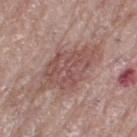Q: Is there a histopathology result?
A: total-body-photography surveillance lesion; no biopsy
Q: How large is the lesion?
A: ≈7 mm
Q: What is the imaging modality?
A: ~15 mm tile from a whole-body skin photo
Q: Automated lesion metrics?
A: an average lesion color of about L≈51 a*≈20 b*≈22 (CIELAB) and about 9 CIELAB-L* units darker than the surrounding skin; border irregularity of about 4.5 on a 0–10 scale, internal color variation of about 3 on a 0–10 scale, and radial color variation of about 1
Q: What lighting was used for the tile?
A: white-light
Q: What are the patient's age and sex?
A: female, approximately 65 years of age
Q: Where on the body is the lesion?
A: the right thigh